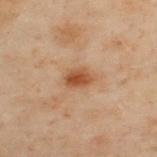Q: Was this lesion biopsied?
A: imaged on a skin check; not biopsied
Q: What kind of image is this?
A: ~15 mm crop, total-body skin-cancer survey
Q: Lesion location?
A: the upper back
Q: What are the patient's age and sex?
A: male, aged around 50